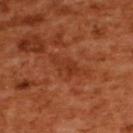Notes:
– biopsy status — catalogued during a skin exam; not biopsied
– subject — female, aged 53 to 57
– image — 15 mm crop, total-body photography
– site — the upper back